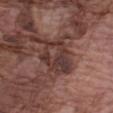| feature | finding |
|---|---|
| follow-up | total-body-photography surveillance lesion; no biopsy |
| lighting | white-light illumination |
| subject | male, aged 73 to 77 |
| image | ~15 mm crop, total-body skin-cancer survey |
| anatomic site | the left forearm |